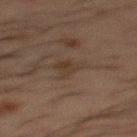Assessment: This lesion was catalogued during total-body skin photography and was not selected for biopsy. Clinical summary: Cropped from a total-body skin-imaging series; the visible field is about 15 mm. From the mid back. A male patient in their 50s.